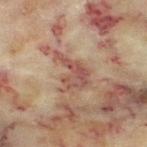{"biopsy_status": "not biopsied; imaged during a skin examination", "lighting": "cross-polarized", "automated_metrics": {"cielab_L": 47, "cielab_a": 20, "cielab_b": 24, "vs_skin_darker_L": 9.0, "vs_skin_contrast_norm": 7.5, "lesion_detection_confidence_0_100": 65}, "patient": {"sex": "female", "age_approx": 60}, "site": "left thigh", "image": {"source": "total-body photography crop", "field_of_view_mm": 15}}